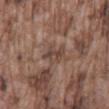site: mid back
lighting: white-light
image:
  source: total-body photography crop
  field_of_view_mm: 15
lesion_size:
  long_diameter_mm_approx: 3.0
patient:
  sex: male
  age_approx: 75